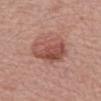The lesion was photographed on a routine skin check and not biopsied; there is no pathology result.